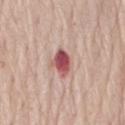{"biopsy_status": "not biopsied; imaged during a skin examination", "image": {"source": "total-body photography crop", "field_of_view_mm": 15}, "lesion_size": {"long_diameter_mm_approx": 3.0}, "site": "lower back", "patient": {"sex": "female", "age_approx": 75}}